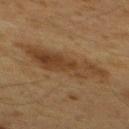Impression: Recorded during total-body skin imaging; not selected for excision or biopsy. Acquisition and patient details: The lesion is on the mid back. A male patient aged 58 to 62. A close-up tile cropped from a whole-body skin photograph, about 15 mm across.